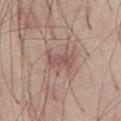<tbp_lesion>
<biopsy_status>not biopsied; imaged during a skin examination</biopsy_status>
<patient>
  <sex>male</sex>
  <age_approx>65</age_approx>
</patient>
<image>
  <source>total-body photography crop</source>
  <field_of_view_mm>15</field_of_view_mm>
</image>
<site>abdomen</site>
</tbp_lesion>